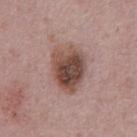Assessment: Imaged during a routine full-body skin examination; the lesion was not biopsied and no histopathology is available. Context: Located on the abdomen. Cropped from a whole-body photographic skin survey; the tile spans about 15 mm. A male subject aged 73–77. Automated tile analysis of the lesion measured a mean CIELAB color near L≈47 a*≈18 b*≈23 and a normalized lesion–skin contrast near 10.5. The software also gave border irregularity of about 2 on a 0–10 scale and internal color variation of about 7.5 on a 0–10 scale. Captured under white-light illumination.